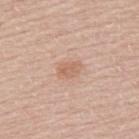Acquisition and patient details:
This image is a 15 mm lesion crop taken from a total-body photograph. A male subject, roughly 50 years of age. Measured at roughly 2.5 mm in maximum diameter. Captured under white-light illumination. Located on the upper back.